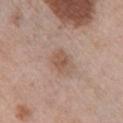Q: Was a biopsy performed?
A: imaged on a skin check; not biopsied
Q: What is the anatomic site?
A: the right upper arm
Q: What are the patient's age and sex?
A: female, in their mid- to late 60s
Q: What kind of image is this?
A: ~15 mm tile from a whole-body skin photo